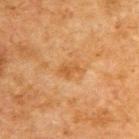Impression: This lesion was catalogued during total-body skin photography and was not selected for biopsy.